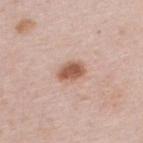| field | value |
|---|---|
| workup | total-body-photography surveillance lesion; no biopsy |
| image source | total-body-photography crop, ~15 mm field of view |
| size | about 3 mm |
| tile lighting | white-light illumination |
| subject | male, in their 40s |
| anatomic site | the upper back |